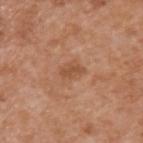biopsy status — total-body-photography surveillance lesion; no biopsy
image source — total-body-photography crop, ~15 mm field of view
subject — male, aged 63–67
anatomic site — the upper back